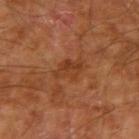Captured during whole-body skin photography for melanoma surveillance; the lesion was not biopsied. Cropped from a whole-body photographic skin survey; the tile spans about 15 mm. Measured at roughly 3.5 mm in maximum diameter. From the right upper arm. The lesion-visualizer software estimated a mean CIELAB color near L≈38 a*≈25 b*≈35. The analysis additionally found lesion-presence confidence of about 100/100. A male subject, aged 68–72. Captured under cross-polarized illumination.